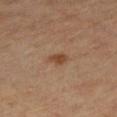workup: total-body-photography surveillance lesion; no biopsy
image source: 15 mm crop, total-body photography
location: the right lower leg
patient: female, aged 63–67
illumination: cross-polarized
automated lesion analysis: a footprint of about 3 mm², an eccentricity of roughly 0.6, and two-axis asymmetry of about 0.3; a color-variation rating of about 1/10
diameter: ~2 mm (longest diameter)